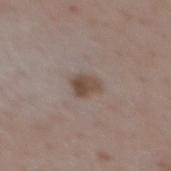Recorded during total-body skin imaging; not selected for excision or biopsy. Imaged with white-light lighting. Cropped from a whole-body photographic skin survey; the tile spans about 15 mm. Measured at roughly 2.5 mm in maximum diameter. From the mid back. Automated tile analysis of the lesion measured an area of roughly 4.5 mm², an outline eccentricity of about 0.65 (0 = round, 1 = elongated), and a shape-asymmetry score of about 0.25 (0 = symmetric). And it measured a nevus-likeness score of about 75/100 and a lesion-detection confidence of about 100/100. The patient is a female aged 43 to 47.